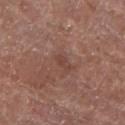Recorded during total-body skin imaging; not selected for excision or biopsy. On the right lower leg. The patient is a female roughly 80 years of age. The tile uses white-light illumination. The recorded lesion diameter is about 2.5 mm. Cropped from a whole-body photographic skin survey; the tile spans about 15 mm.